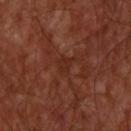  biopsy_status: not biopsied; imaged during a skin examination
  image:
    source: total-body photography crop
    field_of_view_mm: 15
  automated_metrics:
    cielab_L: 27
    cielab_a: 24
    cielab_b: 27
    vs_skin_darker_L: 4.0
    vs_skin_contrast_norm: 4.5
    border_irregularity_0_10: 5.0
    color_variation_0_10: 1.5
    peripheral_color_asymmetry: 0.5
    nevus_likeness_0_100: 0
    lesion_detection_confidence_0_100: 95
  lighting: cross-polarized
  lesion_size:
    long_diameter_mm_approx: 2.5
  patient:
    sex: male
    age_approx: 55
  site: upper back